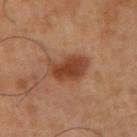Q: Is there a histopathology result?
A: imaged on a skin check; not biopsied
Q: How was the tile lit?
A: cross-polarized illumination
Q: What are the patient's age and sex?
A: male, aged 48 to 52
Q: Lesion location?
A: the right upper arm
Q: How was this image acquired?
A: ~15 mm tile from a whole-body skin photo
Q: What is the lesion's diameter?
A: ~4 mm (longest diameter)